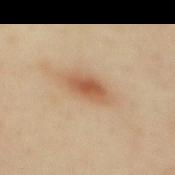Q: Was a biopsy performed?
A: no biopsy performed (imaged during a skin exam)
Q: How was this image acquired?
A: ~15 mm crop, total-body skin-cancer survey
Q: Automated lesion metrics?
A: a mean CIELAB color near L≈58 a*≈22 b*≈36, roughly 12 lightness units darker than nearby skin, and a normalized lesion–skin contrast near 8; an automated nevus-likeness rating near 95 out of 100 and a lesion-detection confidence of about 100/100
Q: What lighting was used for the tile?
A: cross-polarized illumination
Q: Who is the patient?
A: female, roughly 40 years of age
Q: Lesion size?
A: ~4 mm (longest diameter)
Q: Where on the body is the lesion?
A: the mid back